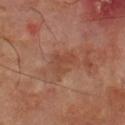| key | value |
|---|---|
| follow-up | imaged on a skin check; not biopsied |
| anatomic site | the left forearm |
| tile lighting | cross-polarized illumination |
| patient | male, in their 70s |
| image | total-body-photography crop, ~15 mm field of view |